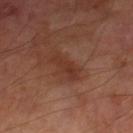From the right lower leg.
Captured under cross-polarized illumination.
Longest diameter approximately 4 mm.
The subject is a male aged around 70.
Cropped from a total-body skin-imaging series; the visible field is about 15 mm.